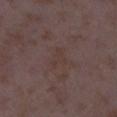| feature | finding |
|---|---|
| biopsy status | catalogued during a skin exam; not biopsied |
| location | the right lower leg |
| subject | female, in their mid- to late 30s |
| tile lighting | white-light illumination |
| TBP lesion metrics | a shape eccentricity near 0.8 and a shape-asymmetry score of about 0.5 (0 = symmetric); a border-irregularity rating of about 5/10 and a color-variation rating of about 1.5/10 |
| image source | total-body-photography crop, ~15 mm field of view |